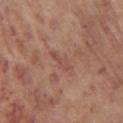notes = catalogued during a skin exam; not biopsied | diameter = about 3 mm | lighting = white-light | body site = the chest | subject = male, aged 53–57 | imaging modality = total-body-photography crop, ~15 mm field of view.